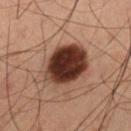Captured during whole-body skin photography for melanoma surveillance; the lesion was not biopsied. An algorithmic analysis of the crop reported an outline eccentricity of about 0.55 (0 = round, 1 = elongated) and a shape-asymmetry score of about 0.15 (0 = symmetric). It also reported a border-irregularity index near 1.5/10, a within-lesion color-variation index near 6/10, and a peripheral color-asymmetry measure near 2. And it measured lesion-presence confidence of about 100/100. Captured under cross-polarized illumination. A roughly 15 mm field-of-view crop from a total-body skin photograph. The lesion is located on the left thigh. A male subject roughly 60 years of age. Longest diameter approximately 6 mm.